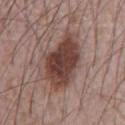Q: Was this lesion biopsied?
A: no biopsy performed (imaged during a skin exam)
Q: Lesion size?
A: about 7 mm
Q: How was the tile lit?
A: white-light illumination
Q: What is the anatomic site?
A: the abdomen
Q: Who is the patient?
A: male, in their 70s
Q: What kind of image is this?
A: ~15 mm crop, total-body skin-cancer survey